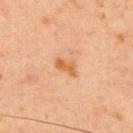notes: no biopsy performed (imaged during a skin exam); lighting: cross-polarized; size: about 3 mm; patient: male, about 65 years old; acquisition: ~15 mm tile from a whole-body skin photo; location: the chest.